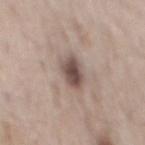Clinical impression: The lesion was photographed on a routine skin check and not biopsied; there is no pathology result. Context: The lesion is located on the mid back. The recorded lesion diameter is about 4 mm. A region of skin cropped from a whole-body photographic capture, roughly 15 mm wide. A male subject aged around 55.